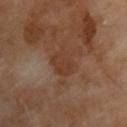Impression:
The lesion was photographed on a routine skin check and not biopsied; there is no pathology result.
Image and clinical context:
A lesion tile, about 15 mm wide, cut from a 3D total-body photograph. The tile uses cross-polarized illumination. Measured at roughly 3 mm in maximum diameter. The lesion is located on the chest. A male subject, about 55 years old.